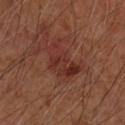This lesion was catalogued during total-body skin photography and was not selected for biopsy.
A male patient aged around 65.
From the left forearm.
Imaged with cross-polarized lighting.
A 15 mm crop from a total-body photograph taken for skin-cancer surveillance.
Measured at roughly 5 mm in maximum diameter.